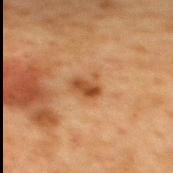Clinical impression: Recorded during total-body skin imaging; not selected for excision or biopsy. Background: Automated image analysis of the tile measured a footprint of about 5 mm², an eccentricity of roughly 0.7, and a shape-asymmetry score of about 0.25 (0 = symmetric). The analysis additionally found border irregularity of about 3 on a 0–10 scale and peripheral color asymmetry of about 1.5. Longest diameter approximately 3 mm. The tile uses cross-polarized illumination. The lesion is located on the back. The subject is a female approximately 40 years of age. A 15 mm close-up extracted from a 3D total-body photography capture.